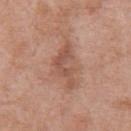The lesion was tiled from a total-body skin photograph and was not biopsied.
Longest diameter approximately 6 mm.
Cropped from a total-body skin-imaging series; the visible field is about 15 mm.
A male patient, aged approximately 70.
The lesion is located on the chest.